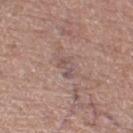| field | value |
|---|---|
| biopsy status | catalogued during a skin exam; not biopsied |
| automated metrics | a lesion area of about 3.5 mm², an eccentricity of roughly 0.85, and a shape-asymmetry score of about 0.35 (0 = symmetric); about 7 CIELAB-L* units darker than the surrounding skin and a lesion-to-skin contrast of about 5.5 (normalized; higher = more distinct); a color-variation rating of about 1/10 and a peripheral color-asymmetry measure near 0; an automated nevus-likeness rating near 0 out of 100 and a lesion-detection confidence of about 70/100 |
| image source | total-body-photography crop, ~15 mm field of view |
| subject | female, aged 58 to 62 |
| body site | the right thigh |
| lighting | white-light illumination |
| size | ~2.5 mm (longest diameter) |